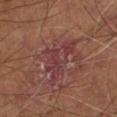Imaged during a routine full-body skin examination; the lesion was not biopsied and no histopathology is available.
The recorded lesion diameter is about 6 mm.
A male patient aged approximately 60.
Captured under cross-polarized illumination.
The total-body-photography lesion software estimated border irregularity of about 7.5 on a 0–10 scale, internal color variation of about 4.5 on a 0–10 scale, and peripheral color asymmetry of about 1.5. It also reported an automated nevus-likeness rating near 0 out of 100.
From the right leg.
A close-up tile cropped from a whole-body skin photograph, about 15 mm across.